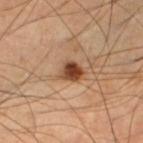<record>
  <biopsy_status>not biopsied; imaged during a skin examination</biopsy_status>
  <patient>
    <sex>male</sex>
    <age_approx>50</age_approx>
  </patient>
  <image>
    <source>total-body photography crop</source>
    <field_of_view_mm>15</field_of_view_mm>
  </image>
  <site>left lower leg</site>
</record>